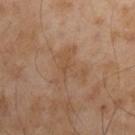Assessment:
Recorded during total-body skin imaging; not selected for excision or biopsy.
Background:
A male subject approximately 55 years of age. Cropped from a whole-body photographic skin survey; the tile spans about 15 mm. Located on the left upper arm.